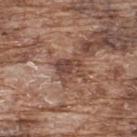Findings:
* biopsy status · catalogued during a skin exam; not biopsied
* lesion diameter · ≈3 mm
* subject · male, aged 73–77
* automated metrics · a lesion color around L≈45 a*≈20 b*≈25 in CIELAB, a lesion–skin lightness drop of about 10, and a normalized border contrast of about 7.5; a nevus-likeness score of about 0/100
* site · the upper back
* image source · total-body-photography crop, ~15 mm field of view